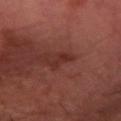The lesion was photographed on a routine skin check and not biopsied; there is no pathology result.
A subject in their mid- to late 60s.
On the right forearm.
A lesion tile, about 15 mm wide, cut from a 3D total-body photograph.
Imaged with cross-polarized lighting.
The lesion's longest dimension is about 2.5 mm.